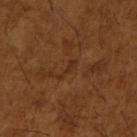Recorded during total-body skin imaging; not selected for excision or biopsy. Cropped from a total-body skin-imaging series; the visible field is about 15 mm. On the right upper arm. Captured under cross-polarized illumination. The lesion's longest dimension is about 3 mm. A male patient, roughly 65 years of age.